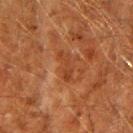This lesion was catalogued during total-body skin photography and was not selected for biopsy.
The patient is a male about 60 years old.
Cropped from a total-body skin-imaging series; the visible field is about 15 mm.
Located on the right upper arm.